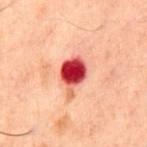This lesion was catalogued during total-body skin photography and was not selected for biopsy.
A male patient roughly 60 years of age.
The lesion is on the chest.
Approximately 4.5 mm at its widest.
The lesion-visualizer software estimated a lesion–skin lightness drop of about 20 and a normalized lesion–skin contrast near 15. It also reported border irregularity of about 3.5 on a 0–10 scale, a color-variation rating of about 4/10, and peripheral color asymmetry of about 1. The analysis additionally found lesion-presence confidence of about 100/100.
A close-up tile cropped from a whole-body skin photograph, about 15 mm across.
Imaged with cross-polarized lighting.